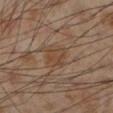The lesion was photographed on a routine skin check and not biopsied; there is no pathology result.
The lesion is located on the left lower leg.
A male patient roughly 55 years of age.
Cropped from a total-body skin-imaging series; the visible field is about 15 mm.